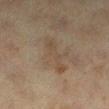Notes:
• follow-up · total-body-photography surveillance lesion; no biopsy
• subject · female, aged around 40
• lighting · cross-polarized illumination
• imaging modality · total-body-photography crop, ~15 mm field of view
• anatomic site · the left lower leg
• diameter · about 5 mm